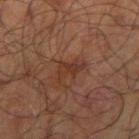<lesion>
<biopsy_status>not biopsied; imaged during a skin examination</biopsy_status>
<automated_metrics>
  <area_mm2_approx>4.5</area_mm2_approx>
  <eccentricity>0.75</eccentricity>
  <vs_skin_darker_L>6.0</vs_skin_darker_L>
  <color_variation_0_10>3.5</color_variation_0_10>
  <peripheral_color_asymmetry>1.5</peripheral_color_asymmetry>
</automated_metrics>
<site>right upper arm</site>
<image>
  <source>total-body photography crop</source>
  <field_of_view_mm>15</field_of_view_mm>
</image>
<patient>
  <sex>male</sex>
  <age_approx>70</age_approx>
</patient>
<lighting>cross-polarized</lighting>
</lesion>